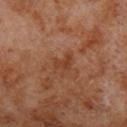Acquisition and patient details:
Automated image analysis of the tile measured a border-irregularity index near 5/10 and radial color variation of about 0.5. The software also gave an automated nevus-likeness rating near 0 out of 100 and a detector confidence of about 100 out of 100 that the crop contains a lesion. A roughly 15 mm field-of-view crop from a total-body skin photograph. A male subject, aged around 70. Captured under cross-polarized illumination. Longest diameter approximately 2.5 mm. From the left lower leg.